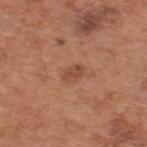Approximately 2.5 mm at its widest.
An algorithmic analysis of the crop reported a footprint of about 4 mm², a shape eccentricity near 0.75, and two-axis asymmetry of about 0.25. The analysis additionally found a mean CIELAB color near L≈48 a*≈24 b*≈30, roughly 8 lightness units darker than nearby skin, and a lesion-to-skin contrast of about 6 (normalized; higher = more distinct).
Cropped from a whole-body photographic skin survey; the tile spans about 15 mm.
This is a white-light tile.
On the back.
A male patient, about 65 years old.